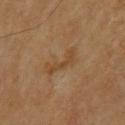notes: no biopsy performed (imaged during a skin exam)